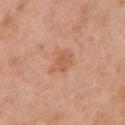Q: Was a biopsy performed?
A: imaged on a skin check; not biopsied
Q: What is the imaging modality?
A: total-body-photography crop, ~15 mm field of view
Q: Lesion location?
A: the left upper arm
Q: What are the patient's age and sex?
A: female, aged around 40